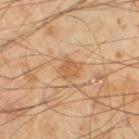workup: imaged on a skin check; not biopsied | lesion size: ≈3 mm | image-analysis metrics: a footprint of about 5.5 mm², a shape eccentricity near 0.4, and a symmetry-axis asymmetry near 0.25; border irregularity of about 2.5 on a 0–10 scale, a within-lesion color-variation index near 2/10, and peripheral color asymmetry of about 0.5; a nevus-likeness score of about 30/100 and a lesion-detection confidence of about 100/100 | subject: male, in their mid- to late 40s | image: ~15 mm tile from a whole-body skin photo | tile lighting: cross-polarized illumination | anatomic site: the left thigh.